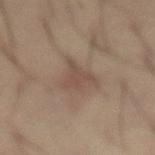Clinical impression: Imaged during a routine full-body skin examination; the lesion was not biopsied and no histopathology is available. Context: A male subject, aged around 60. The lesion is on the abdomen. Cropped from a whole-body photographic skin survey; the tile spans about 15 mm. The tile uses cross-polarized illumination. About 4 mm across.